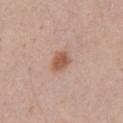image source: ~15 mm crop, total-body skin-cancer survey
location: the chest
tile lighting: white-light illumination
subject: male, aged 33 to 37